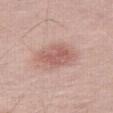Q: Is there a histopathology result?
A: total-body-photography surveillance lesion; no biopsy
Q: How large is the lesion?
A: ≈4.5 mm
Q: What is the imaging modality?
A: ~15 mm tile from a whole-body skin photo
Q: Patient demographics?
A: female, aged 58 to 62
Q: Lesion location?
A: the leg